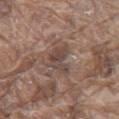This lesion was catalogued during total-body skin photography and was not selected for biopsy.
On the chest.
Longest diameter approximately 4 mm.
Captured under white-light illumination.
The patient is a male aged around 80.
A 15 mm crop from a total-body photograph taken for skin-cancer surveillance.